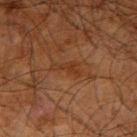Notes:
- location: the arm
- acquisition: total-body-photography crop, ~15 mm field of view
- illumination: cross-polarized illumination
- subject: male, roughly 65 years of age